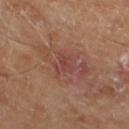lesion diameter: ~3.5 mm (longest diameter)
automated lesion analysis: a footprint of about 5.5 mm² and a shape-asymmetry score of about 0.35 (0 = symmetric); a lesion color around L≈43 a*≈24 b*≈24 in CIELAB and about 6 CIELAB-L* units darker than the surrounding skin; a classifier nevus-likeness of about 0/100
lighting: cross-polarized illumination
patient: male, aged 63 to 67
site: the leg
image: 15 mm crop, total-body photography